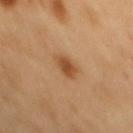workup = imaged on a skin check; not biopsied | acquisition = total-body-photography crop, ~15 mm field of view | lighting = cross-polarized | lesion size = about 2.5 mm | anatomic site = the mid back | patient = male, approximately 50 years of age.